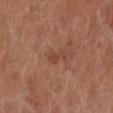Captured during whole-body skin photography for melanoma surveillance; the lesion was not biopsied.
The lesion is on the left leg.
A roughly 15 mm field-of-view crop from a total-body skin photograph.
The subject is a female aged 63 to 67.